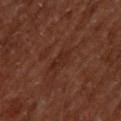imaging modality: 15 mm crop, total-body photography
anatomic site: the upper back
subject: male, in their mid- to late 60s